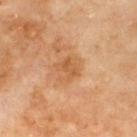Imaged during a routine full-body skin examination; the lesion was not biopsied and no histopathology is available. The subject is a male aged approximately 70. The recorded lesion diameter is about 3 mm. The total-body-photography lesion software estimated an area of roughly 5.5 mm², an outline eccentricity of about 0.3 (0 = round, 1 = elongated), and two-axis asymmetry of about 0.35. The analysis additionally found a border-irregularity index near 4.5/10, a within-lesion color-variation index near 3/10, and a peripheral color-asymmetry measure near 1. The analysis additionally found an automated nevus-likeness rating near 0 out of 100. Captured under cross-polarized illumination. On the upper back. A 15 mm close-up extracted from a 3D total-body photography capture.